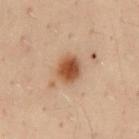This lesion was catalogued during total-body skin photography and was not selected for biopsy. The tile uses cross-polarized illumination. The lesion is on the mid back. The patient is a male aged 48–52. A 15 mm close-up extracted from a 3D total-body photography capture.